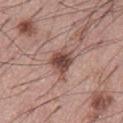biopsy_status: not biopsied; imaged during a skin examination
lighting: white-light
patient:
  sex: male
  age_approx: 55
site: abdomen
image:
  source: total-body photography crop
  field_of_view_mm: 15